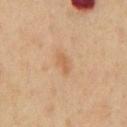| feature | finding |
|---|---|
| notes | total-body-photography surveillance lesion; no biopsy |